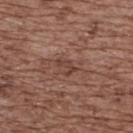The lesion was tiled from a total-body skin photograph and was not biopsied. A female subject roughly 75 years of age. Measured at roughly 2.5 mm in maximum diameter. A roughly 15 mm field-of-view crop from a total-body skin photograph. The lesion is located on the upper back.